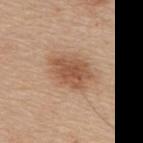Q: Was this lesion biopsied?
A: no biopsy performed (imaged during a skin exam)
Q: Where on the body is the lesion?
A: the upper back
Q: How was this image acquired?
A: ~15 mm tile from a whole-body skin photo
Q: Who is the patient?
A: male, roughly 65 years of age